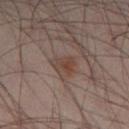workup: total-body-photography surveillance lesion; no biopsy | tile lighting: cross-polarized | patient: male, aged 38 to 42 | automated lesion analysis: a lesion area of about 4.5 mm² and an outline eccentricity of about 0.7 (0 = round, 1 = elongated); a border-irregularity index near 4/10, a within-lesion color-variation index near 4/10, and peripheral color asymmetry of about 1 | acquisition: total-body-photography crop, ~15 mm field of view | diameter: about 3 mm | site: the leg.